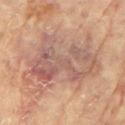{
  "biopsy_status": "not biopsied; imaged during a skin examination",
  "lesion_size": {
    "long_diameter_mm_approx": 9.5
  },
  "patient": {
    "sex": "female",
    "age_approx": 80
  },
  "lighting": "cross-polarized",
  "image": {
    "source": "total-body photography crop",
    "field_of_view_mm": 15
  },
  "automated_metrics": {
    "border_irregularity_0_10": 7.5,
    "color_variation_0_10": 6.5,
    "peripheral_color_asymmetry": 2.0
  },
  "site": "right arm"
}